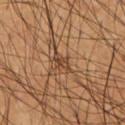Case summary:
* site — the chest
* patient — male, roughly 55 years of age
* image-analysis metrics — a lesion area of about 3.5 mm², an eccentricity of roughly 0.85, and a symmetry-axis asymmetry near 0.35; border irregularity of about 4.5 on a 0–10 scale, internal color variation of about 1.5 on a 0–10 scale, and a peripheral color-asymmetry measure near 0.5; a nevus-likeness score of about 0/100 and a lesion-detection confidence of about 0/100
* lesion diameter — ~3 mm (longest diameter)
* illumination — cross-polarized
* acquisition — ~15 mm crop, total-body skin-cancer survey